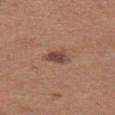Impression: Part of a total-body skin-imaging series; this lesion was reviewed on a skin check and was not flagged for biopsy. Acquisition and patient details: The lesion is located on the left thigh. A close-up tile cropped from a whole-body skin photograph, about 15 mm across. A female subject aged 38–42. Measured at roughly 3 mm in maximum diameter.